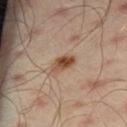Assessment:
Imaged during a routine full-body skin examination; the lesion was not biopsied and no histopathology is available.
Clinical summary:
A male patient, aged 43 to 47. From the right thigh. A close-up tile cropped from a whole-body skin photograph, about 15 mm across. Longest diameter approximately 3 mm. Imaged with cross-polarized lighting.